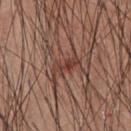No biopsy was performed on this lesion — it was imaged during a full skin examination and was not determined to be concerning. A male patient aged 58–62. A close-up tile cropped from a whole-body skin photograph, about 15 mm across. The lesion is located on the chest. The lesion-visualizer software estimated a lesion area of about 5 mm², a shape eccentricity near 0.9, and a symmetry-axis asymmetry near 0.35. The analysis additionally found border irregularity of about 5 on a 0–10 scale, a color-variation rating of about 2/10, and a peripheral color-asymmetry measure near 0.5. It also reported a detector confidence of about 100 out of 100 that the crop contains a lesion. Longest diameter approximately 3.5 mm. Captured under white-light illumination.